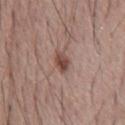The lesion was tiled from a total-body skin photograph and was not biopsied.
The tile uses white-light illumination.
The lesion is on the chest.
A roughly 15 mm field-of-view crop from a total-body skin photograph.
A male subject, aged around 65.
The lesion's longest dimension is about 2.5 mm.